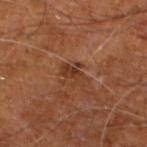The lesion was tiled from a total-body skin photograph and was not biopsied. The lesion is located on the right leg. A 15 mm close-up tile from a total-body photography series done for melanoma screening. The subject is a male approximately 60 years of age. About 3 mm across.